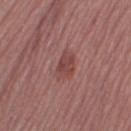Impression: No biopsy was performed on this lesion — it was imaged during a full skin examination and was not determined to be concerning. Acquisition and patient details: Cropped from a whole-body photographic skin survey; the tile spans about 15 mm. The lesion is located on the right thigh. The tile uses white-light illumination. A female subject aged approximately 55.